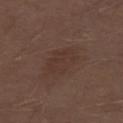{
  "biopsy_status": "not biopsied; imaged during a skin examination",
  "site": "right thigh",
  "lighting": "white-light",
  "patient": {
    "sex": "male",
    "age_approx": 70
  },
  "automated_metrics": {
    "area_mm2_approx": 9.5,
    "eccentricity": 0.75,
    "shape_asymmetry": 0.15,
    "nevus_likeness_0_100": 5,
    "lesion_detection_confidence_0_100": 100
  },
  "image": {
    "source": "total-body photography crop",
    "field_of_view_mm": 15
  }
}